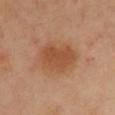subject: female, approximately 40 years of age; site: the chest; diameter: ≈5 mm; imaging modality: ~15 mm tile from a whole-body skin photo.